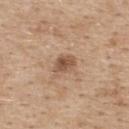subject: female, aged around 45
lighting: white-light
automated lesion analysis: a footprint of about 5 mm² and an outline eccentricity of about 0.65 (0 = round, 1 = elongated); a lesion color around L≈53 a*≈18 b*≈30 in CIELAB, roughly 11 lightness units darker than nearby skin, and a lesion-to-skin contrast of about 7.5 (normalized; higher = more distinct); a border-irregularity index near 2/10
lesion size: ~3 mm (longest diameter)
acquisition: total-body-photography crop, ~15 mm field of view
location: the upper back